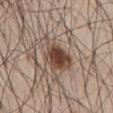Notes:
* biopsy status: no biopsy performed (imaged during a skin exam)
* image source: total-body-photography crop, ~15 mm field of view
* site: the abdomen
* patient: male, roughly 60 years of age
* size: ≈5.5 mm
* lighting: white-light